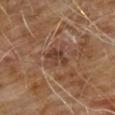Findings:
– notes: catalogued during a skin exam; not biopsied
– automated lesion analysis: a footprint of about 4.5 mm²; an average lesion color of about L≈37 a*≈19 b*≈26 (CIELAB), roughly 8 lightness units darker than nearby skin, and a lesion-to-skin contrast of about 7.5 (normalized; higher = more distinct); a color-variation rating of about 2/10 and a peripheral color-asymmetry measure near 0.5; a classifier nevus-likeness of about 0/100 and lesion-presence confidence of about 100/100
– patient: male, aged around 60
– imaging modality: total-body-photography crop, ~15 mm field of view
– body site: the chest
– lighting: cross-polarized
– lesion size: about 2.5 mm